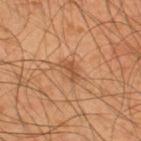Impression:
No biopsy was performed on this lesion — it was imaged during a full skin examination and was not determined to be concerning.
Background:
The lesion is on the upper back. An algorithmic analysis of the crop reported a lesion color around L≈48 a*≈22 b*≈35 in CIELAB and about 9 CIELAB-L* units darker than the surrounding skin. The analysis additionally found an automated nevus-likeness rating near 50 out of 100 and a detector confidence of about 100 out of 100 that the crop contains a lesion. Captured under cross-polarized illumination. A male patient, approximately 45 years of age. A roughly 15 mm field-of-view crop from a total-body skin photograph. Longest diameter approximately 2.5 mm.